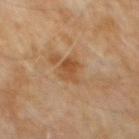Recorded during total-body skin imaging; not selected for excision or biopsy.
A male subject in their 60s.
Cropped from a whole-body photographic skin survey; the tile spans about 15 mm.
An algorithmic analysis of the crop reported border irregularity of about 3 on a 0–10 scale and a color-variation rating of about 3/10.
Measured at roughly 3 mm in maximum diameter.
On the abdomen.